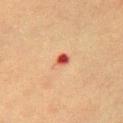automated metrics: a shape eccentricity near 0.75 and two-axis asymmetry of about 0.25; subject: female, approximately 55 years of age; anatomic site: the front of the torso; lighting: cross-polarized; image source: 15 mm crop, total-body photography; size: about 2.5 mm.